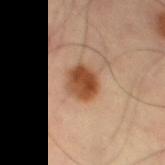Notes:
- notes — catalogued during a skin exam; not biopsied
- size — ~3.5 mm (longest diameter)
- automated metrics — a border-irregularity index near 1.5/10, a within-lesion color-variation index near 6/10, and peripheral color asymmetry of about 2; a lesion-detection confidence of about 100/100
- site — the left thigh
- imaging modality — ~15 mm tile from a whole-body skin photo
- illumination — cross-polarized